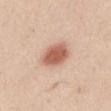Case summary:
* notes — total-body-photography surveillance lesion; no biopsy
* lighting — white-light
* lesion diameter — about 4 mm
* patient — male, in their mid- to late 20s
* imaging modality — 15 mm crop, total-body photography
* image-analysis metrics — a shape-asymmetry score of about 0.15 (0 = symmetric); a lesion color around L≈60 a*≈23 b*≈30 in CIELAB, roughly 15 lightness units darker than nearby skin, and a normalized border contrast of about 9; a border-irregularity rating of about 1.5/10 and a peripheral color-asymmetry measure near 1; an automated nevus-likeness rating near 100 out of 100 and a detector confidence of about 100 out of 100 that the crop contains a lesion
* site — the mid back